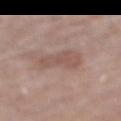Clinical impression: Imaged during a routine full-body skin examination; the lesion was not biopsied and no histopathology is available. Acquisition and patient details: Imaged with white-light lighting. A female subject, aged 73–77. The lesion's longest dimension is about 4 mm. From the right lower leg. A region of skin cropped from a whole-body photographic capture, roughly 15 mm wide. The lesion-visualizer software estimated a border-irregularity rating of about 3.5/10, a within-lesion color-variation index near 2/10, and a peripheral color-asymmetry measure near 1. And it measured an automated nevus-likeness rating near 0 out of 100 and a lesion-detection confidence of about 100/100.